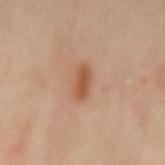Findings:
* subject — female, about 60 years old
* image source — 15 mm crop, total-body photography
* body site — the mid back
* lighting — cross-polarized illumination
* lesion diameter — about 3.5 mm